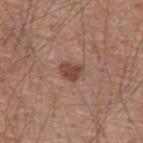The lesion was photographed on a routine skin check and not biopsied; there is no pathology result. Imaged with white-light lighting. A roughly 15 mm field-of-view crop from a total-body skin photograph. Approximately 3 mm at its widest. A male subject aged 53–57. The total-body-photography lesion software estimated a lesion–skin lightness drop of about 11. And it measured border irregularity of about 2.5 on a 0–10 scale and a color-variation rating of about 2/10. The lesion is located on the mid back.